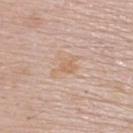Assessment:
This lesion was catalogued during total-body skin photography and was not selected for biopsy.
Background:
An algorithmic analysis of the crop reported a footprint of about 4.5 mm², a shape eccentricity near 0.85, and two-axis asymmetry of about 0.4. It also reported a lesion-to-skin contrast of about 5.5 (normalized; higher = more distinct). A male patient aged approximately 70. A lesion tile, about 15 mm wide, cut from a 3D total-body photograph. This is a white-light tile. The lesion is located on the back. Approximately 3 mm at its widest.